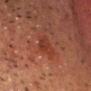<case>
  <biopsy_status>not biopsied; imaged during a skin examination</biopsy_status>
  <lighting>cross-polarized</lighting>
  <site>head or neck</site>
  <lesion_size>
    <long_diameter_mm_approx>3.0</long_diameter_mm_approx>
  </lesion_size>
  <patient>
    <sex>male</sex>
    <age_approx>50</age_approx>
  </patient>
  <image>
    <source>total-body photography crop</source>
    <field_of_view_mm>15</field_of_view_mm>
  </image>
</case>